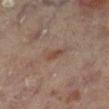Recorded during total-body skin imaging; not selected for excision or biopsy. The patient is a male in their mid- to late 60s. A region of skin cropped from a whole-body photographic capture, roughly 15 mm wide. The lesion is located on the leg.